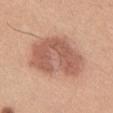No biopsy was performed on this lesion — it was imaged during a full skin examination and was not determined to be concerning.
Located on the right thigh.
A female subject about 55 years old.
A close-up tile cropped from a whole-body skin photograph, about 15 mm across.
The recorded lesion diameter is about 8 mm.